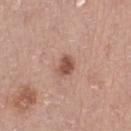  biopsy_status: not biopsied; imaged during a skin examination
  automated_metrics:
    area_mm2_approx: 4.5
    eccentricity: 0.6
    shape_asymmetry: 0.25
    vs_skin_contrast_norm: 8.5
    border_irregularity_0_10: 2.0
    color_variation_0_10: 2.0
    peripheral_color_asymmetry: 0.5
    nevus_likeness_0_100: 80
  lesion_size:
    long_diameter_mm_approx: 2.5
  patient:
    sex: female
    age_approx: 65
  site: right thigh
  lighting: white-light
  image:
    source: total-body photography crop
    field_of_view_mm: 15